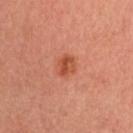{"biopsy_status": "not biopsied; imaged during a skin examination", "lesion_size": {"long_diameter_mm_approx": 2.5}, "site": "head or neck", "automated_metrics": {"border_irregularity_0_10": 2.0, "color_variation_0_10": 4.5, "peripheral_color_asymmetry": 1.5}, "image": {"source": "total-body photography crop", "field_of_view_mm": 15}, "lighting": "cross-polarized", "patient": {"sex": "female", "age_approx": 35}}